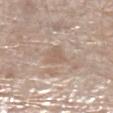Imaged during a routine full-body skin examination; the lesion was not biopsied and no histopathology is available.
A roughly 15 mm field-of-view crop from a total-body skin photograph.
Located on the leg.
A male subject aged 68 to 72.
Longest diameter approximately 2.5 mm.
Imaged with white-light lighting.
An algorithmic analysis of the crop reported roughly 7 lightness units darker than nearby skin and a normalized lesion–skin contrast near 4.5. The software also gave a nevus-likeness score of about 0/100.